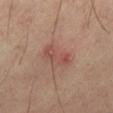biopsy_status: not biopsied; imaged during a skin examination
patient:
  sex: male
  age_approx: 60
site: left thigh
image:
  source: total-body photography crop
  field_of_view_mm: 15